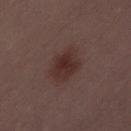Clinical impression: The lesion was photographed on a routine skin check and not biopsied; there is no pathology result. Image and clinical context: From the lower back. The patient is a female approximately 30 years of age. Cropped from a total-body skin-imaging series; the visible field is about 15 mm.